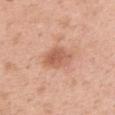Image and clinical context:
A female patient aged 48 to 52. The lesion is located on the upper back. A close-up tile cropped from a whole-body skin photograph, about 15 mm across. The tile uses white-light illumination.